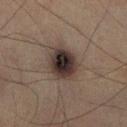{"patient": {"sex": "male", "age_approx": 75}, "site": "right lower leg", "image": {"source": "total-body photography crop", "field_of_view_mm": 15}, "lighting": "cross-polarized"}